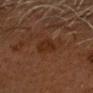Impression:
Recorded during total-body skin imaging; not selected for excision or biopsy.
Acquisition and patient details:
Cropped from a whole-body photographic skin survey; the tile spans about 15 mm. The total-body-photography lesion software estimated a footprint of about 5 mm², an outline eccentricity of about 0.6 (0 = round, 1 = elongated), and a symmetry-axis asymmetry near 0.3. And it measured an average lesion color of about L≈22 a*≈17 b*≈24 (CIELAB) and a normalized border contrast of about 7. And it measured a border-irregularity rating of about 3/10, a within-lesion color-variation index near 1.5/10, and radial color variation of about 0.5. It also reported an automated nevus-likeness rating near 10 out of 100 and a lesion-detection confidence of about 100/100. The patient is a male roughly 60 years of age. The lesion is on the head or neck. This is a cross-polarized tile.